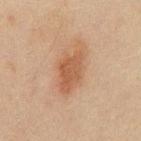Q: What kind of image is this?
A: ~15 mm tile from a whole-body skin photo
Q: Automated lesion metrics?
A: a lesion-to-skin contrast of about 7 (normalized; higher = more distinct); a classifier nevus-likeness of about 90/100 and a detector confidence of about 100 out of 100 that the crop contains a lesion
Q: Illumination type?
A: cross-polarized
Q: How large is the lesion?
A: ~6 mm (longest diameter)
Q: Who is the patient?
A: male, aged approximately 45
Q: Where on the body is the lesion?
A: the abdomen